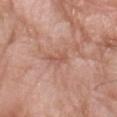workup: imaged on a skin check; not biopsied | size: ~3 mm (longest diameter) | subject: male, aged 58–62 | anatomic site: the left forearm | automated metrics: an area of roughly 3 mm² and two-axis asymmetry of about 0.4; an average lesion color of about L≈55 a*≈24 b*≈29 (CIELAB); a nevus-likeness score of about 0/100 and a detector confidence of about 95 out of 100 that the crop contains a lesion | acquisition: ~15 mm crop, total-body skin-cancer survey.